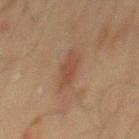| field | value |
|---|---|
| notes | imaged on a skin check; not biopsied |
| image-analysis metrics | a lesion-to-skin contrast of about 6 (normalized; higher = more distinct); a border-irregularity rating of about 4.5/10, a color-variation rating of about 2/10, and radial color variation of about 0.5; a classifier nevus-likeness of about 70/100 |
| tile lighting | cross-polarized |
| lesion diameter | ~4.5 mm (longest diameter) |
| body site | the back |
| patient | male, about 70 years old |
| image source | ~15 mm crop, total-body skin-cancer survey |